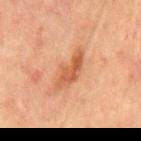lesion size=~5.5 mm (longest diameter)
anatomic site=the chest
image=~15 mm tile from a whole-body skin photo
patient=male, roughly 65 years of age